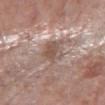  biopsy_status: not biopsied; imaged during a skin examination
  image:
    source: total-body photography crop
    field_of_view_mm: 15
  site: right forearm
  patient:
    sex: female
    age_approx: 70